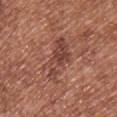Captured during whole-body skin photography for melanoma surveillance; the lesion was not biopsied. This image is a 15 mm lesion crop taken from a total-body photograph. Automated image analysis of the tile measured a border-irregularity rating of about 6.5/10 and a color-variation rating of about 4/10. The lesion is located on the chest. The recorded lesion diameter is about 5.5 mm. A female subject, aged around 40.